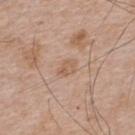Q: Was this lesion biopsied?
A: catalogued during a skin exam; not biopsied
Q: How large is the lesion?
A: about 2.5 mm
Q: How was this image acquired?
A: total-body-photography crop, ~15 mm field of view
Q: What are the patient's age and sex?
A: male, aged 63–67
Q: What is the anatomic site?
A: the upper back